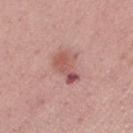workup: total-body-photography surveillance lesion; no biopsy
automated metrics: a shape eccentricity near 0.85 and two-axis asymmetry of about 0.45; about 11 CIELAB-L* units darker than the surrounding skin; border irregularity of about 5 on a 0–10 scale and internal color variation of about 6 on a 0–10 scale; a nevus-likeness score of about 10/100 and lesion-presence confidence of about 100/100
patient: female, about 55 years old
imaging modality: ~15 mm crop, total-body skin-cancer survey
site: the right thigh
lighting: white-light illumination